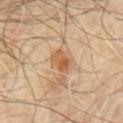The lesion was photographed on a routine skin check and not biopsied; there is no pathology result. Longest diameter approximately 2.5 mm. A male patient, aged 68–72. Located on the chest. Captured under cross-polarized illumination. A 15 mm close-up extracted from a 3D total-body photography capture.